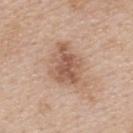biopsy status — imaged on a skin check; not biopsied
image source — ~15 mm crop, total-body skin-cancer survey
automated lesion analysis — a footprint of about 13 mm², an outline eccentricity of about 0.75 (0 = round, 1 = elongated), and a shape-asymmetry score of about 0.3 (0 = symmetric); border irregularity of about 3.5 on a 0–10 scale and radial color variation of about 1.5
subject — female, in their mid- to late 40s
body site — the upper back
lesion size — ~5.5 mm (longest diameter)